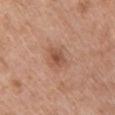Notes:
- workup — no biopsy performed (imaged during a skin exam)
- patient — male, about 65 years old
- image source — 15 mm crop, total-body photography
- body site — the chest